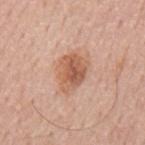workup=total-body-photography surveillance lesion; no biopsy
imaging modality=total-body-photography crop, ~15 mm field of view
patient=male, aged 53 to 57
diameter=about 5 mm
location=the back